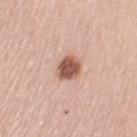Context:
The patient is a female aged 58–62. A 15 mm crop from a total-body photograph taken for skin-cancer surveillance. The lesion is located on the left upper arm.